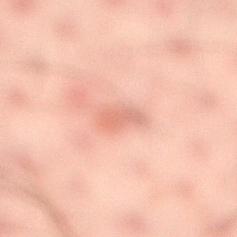Captured during whole-body skin photography for melanoma surveillance; the lesion was not biopsied. A male subject aged around 40. The lesion is on the right lower leg. Approximately 3.5 mm at its widest. A close-up tile cropped from a whole-body skin photograph, about 15 mm across.